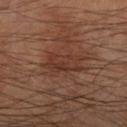Notes:
* biopsy status: total-body-photography surveillance lesion; no biopsy
* lighting: cross-polarized illumination
* automated metrics: an outline eccentricity of about 0.85 (0 = round, 1 = elongated); a mean CIELAB color near L≈33 a*≈20 b*≈25, about 6 CIELAB-L* units darker than the surrounding skin, and a normalized lesion–skin contrast near 6; a border-irregularity index near 4/10, a within-lesion color-variation index near 3.5/10, and radial color variation of about 1.5; a classifier nevus-likeness of about 15/100 and lesion-presence confidence of about 100/100
* site: the right forearm
* imaging modality: ~15 mm tile from a whole-body skin photo
* subject: male, in their mid- to late 60s
* size: about 5 mm